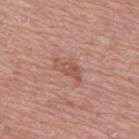Q: Is there a histopathology result?
A: imaged on a skin check; not biopsied
Q: Lesion location?
A: the upper back
Q: How large is the lesion?
A: about 4 mm
Q: Patient demographics?
A: male, roughly 70 years of age
Q: Illumination type?
A: white-light illumination
Q: What kind of image is this?
A: ~15 mm crop, total-body skin-cancer survey